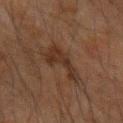Captured during whole-body skin photography for melanoma surveillance; the lesion was not biopsied.
This image is a 15 mm lesion crop taken from a total-body photograph.
Located on the arm.
A male subject aged around 60.
Captured under cross-polarized illumination.
Approximately 5 mm at its widest.